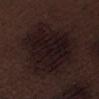notes: catalogued during a skin exam; not biopsied | image source: total-body-photography crop, ~15 mm field of view | lesion size: ~10 mm (longest diameter) | lighting: white-light illumination | patient: male, aged around 70 | body site: the right thigh.